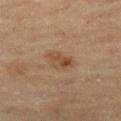Recorded during total-body skin imaging; not selected for excision or biopsy.
A 15 mm close-up tile from a total-body photography series done for melanoma screening.
Longest diameter approximately 3.5 mm.
Imaged with cross-polarized lighting.
From the left thigh.
The patient is a female in their 60s.